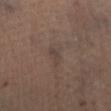Clinical impression:
Imaged during a routine full-body skin examination; the lesion was not biopsied and no histopathology is available.
Background:
A male subject about 50 years old. The lesion is located on the left leg. A 15 mm close-up extracted from a 3D total-body photography capture.